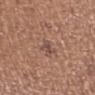follow-up=total-body-photography surveillance lesion; no biopsy
subject=female, aged 48–52
body site=the right upper arm
image=~15 mm tile from a whole-body skin photo